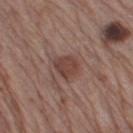The lesion was photographed on a routine skin check and not biopsied; there is no pathology result. Measured at roughly 3 mm in maximum diameter. The subject is a male aged 63–67. A 15 mm close-up extracted from a 3D total-body photography capture. The tile uses white-light illumination. An algorithmic analysis of the crop reported an average lesion color of about L≈42 a*≈20 b*≈23 (CIELAB) and a normalized border contrast of about 7.5. And it measured an automated nevus-likeness rating near 40 out of 100. From the right thigh.